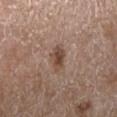Q: Where on the body is the lesion?
A: the right lower leg
Q: How was this image acquired?
A: ~15 mm crop, total-body skin-cancer survey
Q: Patient demographics?
A: female, in their 70s
Q: What is the lesion's diameter?
A: about 3 mm